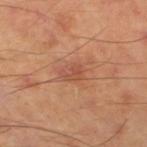Clinical impression: Captured during whole-body skin photography for melanoma surveillance; the lesion was not biopsied. Background: The total-body-photography lesion software estimated a lesion area of about 3.5 mm², a shape eccentricity near 0.75, and a shape-asymmetry score of about 0.45 (0 = symmetric). The software also gave a lesion-detection confidence of about 100/100. A male patient, aged 68 to 72. This image is a 15 mm lesion crop taken from a total-body photograph. The tile uses cross-polarized illumination. Located on the left thigh.